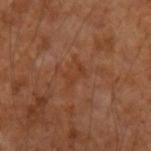No biopsy was performed on this lesion — it was imaged during a full skin examination and was not determined to be concerning.
Longest diameter approximately 2.5 mm.
A lesion tile, about 15 mm wide, cut from a 3D total-body photograph.
The patient is a male aged 53 to 57.
Imaged with cross-polarized lighting.
From the left forearm.